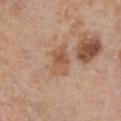The lesion was tiled from a total-body skin photograph and was not biopsied. The lesion is located on the chest. The tile uses white-light illumination. A female subject, about 75 years old. The recorded lesion diameter is about 4 mm. A close-up tile cropped from a whole-body skin photograph, about 15 mm across.